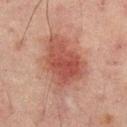lesion size=~6.5 mm (longest diameter)
patient=male, roughly 65 years of age
location=the mid back
TBP lesion metrics=an area of roughly 22 mm², a shape eccentricity near 0.7, and two-axis asymmetry of about 0.3; radial color variation of about 1; a lesion-detection confidence of about 100/100
image source=~15 mm crop, total-body skin-cancer survey
tile lighting=cross-polarized illumination